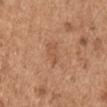biopsy status — no biopsy performed (imaged during a skin exam)
patient — male, about 65 years old
site — the arm
image — ~15 mm tile from a whole-body skin photo
lighting — white-light illumination
automated metrics — a mean CIELAB color near L≈54 a*≈22 b*≈33, about 6 CIELAB-L* units darker than the surrounding skin, and a normalized border contrast of about 4.5; border irregularity of about 4 on a 0–10 scale and peripheral color asymmetry of about 0.5
size — ≈2.5 mm